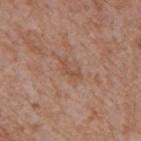Notes:
– biopsy status — total-body-photography surveillance lesion; no biopsy
– lighting — white-light illumination
– automated metrics — roughly 7 lightness units darker than nearby skin and a normalized border contrast of about 5.5; a color-variation rating of about 0/10
– site — the mid back
– subject — male, aged 63 to 67
– image — 15 mm crop, total-body photography